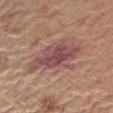notes: no biopsy performed (imaged during a skin exam) | tile lighting: white-light illumination | diameter: about 8 mm | subject: male, aged approximately 70 | image-analysis metrics: an area of roughly 19 mm², a shape eccentricity near 0.9, and two-axis asymmetry of about 0.3; a mean CIELAB color near L≈49 a*≈22 b*≈21, roughly 10 lightness units darker than nearby skin, and a normalized lesion–skin contrast near 9; a border-irregularity index near 5/10, internal color variation of about 5 on a 0–10 scale, and peripheral color asymmetry of about 1.5; a nevus-likeness score of about 5/100 | acquisition: ~15 mm tile from a whole-body skin photo | anatomic site: the right upper arm.